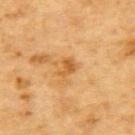From the back.
Cropped from a whole-body photographic skin survey; the tile spans about 15 mm.
Imaged with cross-polarized lighting.
The patient is a male about 85 years old or older.
The lesion's longest dimension is about 2.5 mm.